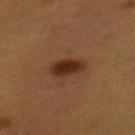The lesion was photographed on a routine skin check and not biopsied; there is no pathology result.
The tile uses cross-polarized illumination.
Located on the mid back.
Cropped from a whole-body photographic skin survey; the tile spans about 15 mm.
Longest diameter approximately 3.5 mm.
A female patient, roughly 40 years of age.